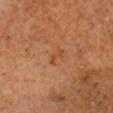Assessment: Recorded during total-body skin imaging; not selected for excision or biopsy. Image and clinical context: The lesion is located on the head or neck. A lesion tile, about 15 mm wide, cut from a 3D total-body photograph. A male subject, aged approximately 60.